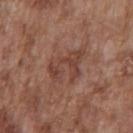Imaged during a routine full-body skin examination; the lesion was not biopsied and no histopathology is available. Measured at roughly 5 mm in maximum diameter. The patient is a male aged around 75. A close-up tile cropped from a whole-body skin photograph, about 15 mm across. An algorithmic analysis of the crop reported an average lesion color of about L≈42 a*≈21 b*≈26 (CIELAB), a lesion–skin lightness drop of about 7, and a normalized border contrast of about 6. It also reported a lesion-detection confidence of about 100/100. Imaged with white-light lighting. On the front of the torso.